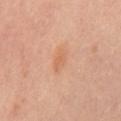biopsy_status: not biopsied; imaged during a skin examination
patient:
  sex: female
  age_approx: 50
image:
  source: total-body photography crop
  field_of_view_mm: 15
site: mid back